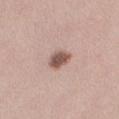Clinical impression: Captured during whole-body skin photography for melanoma surveillance; the lesion was not biopsied. Context: A region of skin cropped from a whole-body photographic capture, roughly 15 mm wide. The total-body-photography lesion software estimated an area of roughly 5.5 mm², a shape eccentricity near 0.45, and two-axis asymmetry of about 0.15. It also reported lesion-presence confidence of about 100/100. The patient is a female aged around 40. The tile uses white-light illumination. The recorded lesion diameter is about 2.5 mm. From the right thigh.